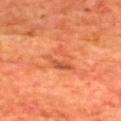Findings:
• notes · imaged on a skin check; not biopsied
• location · the upper back
• image · ~15 mm crop, total-body skin-cancer survey
• subject · male, aged approximately 65
• lesion size · ~3.5 mm (longest diameter)
• illumination · cross-polarized